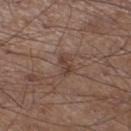notes: catalogued during a skin exam; not biopsied | image: total-body-photography crop, ~15 mm field of view | lighting: white-light illumination | anatomic site: the left lower leg | TBP lesion metrics: a nevus-likeness score of about 0/100 | subject: male, approximately 55 years of age.